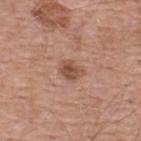biopsy_status: not biopsied; imaged during a skin examination
lighting: white-light
patient:
  sex: male
  age_approx: 55
image:
  source: total-body photography crop
  field_of_view_mm: 15
automated_metrics:
  area_mm2_approx: 4.0
  eccentricity: 0.65
  shape_asymmetry: 0.2
  cielab_L: 50
  cielab_a: 23
  cielab_b: 28
  vs_skin_darker_L: 11.0
  vs_skin_contrast_norm: 8.0
site: back
lesion_size:
  long_diameter_mm_approx: 2.5